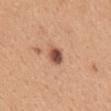<case>
  <biopsy_status>not biopsied; imaged during a skin examination</biopsy_status>
  <image>
    <source>total-body photography crop</source>
    <field_of_view_mm>15</field_of_view_mm>
  </image>
  <site>mid back</site>
  <patient>
    <sex>female</sex>
    <age_approx>30</age_approx>
  </patient>
  <automated_metrics>
    <area_mm2_approx>4.0</area_mm2_approx>
    <eccentricity>0.65</eccentricity>
    <shape_asymmetry>0.2</shape_asymmetry>
    <cielab_L>51</cielab_L>
    <cielab_a>23</cielab_a>
    <cielab_b>30</cielab_b>
    <vs_skin_darker_L>17.0</vs_skin_darker_L>
    <nevus_likeness_0_100>90</nevus_likeness_0_100>
    <lesion_detection_confidence_0_100>100</lesion_detection_confidence_0_100>
  </automated_metrics>
  <lighting>white-light</lighting>
</case>